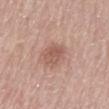follow-up = catalogued during a skin exam; not biopsied | anatomic site = the mid back | automated metrics = a shape eccentricity near 0.8 and a symmetry-axis asymmetry near 0.2; a border-irregularity index near 2/10 | lesion size = ~4 mm (longest diameter) | image = 15 mm crop, total-body photography | patient = male, about 80 years old.